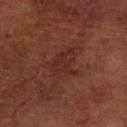{"image": {"source": "total-body photography crop", "field_of_view_mm": 15}, "patient": {"sex": "male", "age_approx": 65}, "site": "left forearm", "automated_metrics": {"area_mm2_approx": 8.5, "shape_asymmetry": 0.35, "cielab_L": 27, "cielab_a": 23, "cielab_b": 23, "vs_skin_darker_L": 5.0, "vs_skin_contrast_norm": 5.0, "border_irregularity_0_10": 4.0, "color_variation_0_10": 2.0, "peripheral_color_asymmetry": 0.5}, "lesion_size": {"long_diameter_mm_approx": 3.5}}